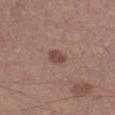workup — imaged on a skin check; not biopsied | patient — male, aged around 55 | image — 15 mm crop, total-body photography | illumination — white-light | automated metrics — a lesion area of about 3.5 mm² and an outline eccentricity of about 0.75 (0 = round, 1 = elongated); a mean CIELAB color near L≈46 a*≈19 b*≈23, a lesion–skin lightness drop of about 10, and a lesion-to-skin contrast of about 8 (normalized; higher = more distinct); border irregularity of about 2.5 on a 0–10 scale and a within-lesion color-variation index near 1.5/10 | body site — the right lower leg.